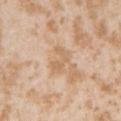The lesion was photographed on a routine skin check and not biopsied; there is no pathology result. The lesion is located on the left upper arm. Automated tile analysis of the lesion measured a classifier nevus-likeness of about 0/100 and a lesion-detection confidence of about 100/100. Approximately 3.5 mm at its widest. The tile uses white-light illumination. A 15 mm crop from a total-body photograph taken for skin-cancer surveillance. The subject is a female aged 23 to 27.